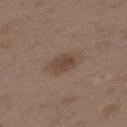  site: upper back
  image:
    source: total-body photography crop
    field_of_view_mm: 15
  patient:
    sex: female
    age_approx: 35
  lighting: white-light